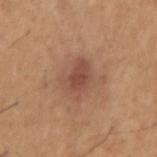{
  "image": {
    "source": "total-body photography crop",
    "field_of_view_mm": 15
  },
  "automated_metrics": {
    "cielab_L": 49,
    "cielab_a": 22,
    "cielab_b": 28,
    "vs_skin_darker_L": 9.0,
    "vs_skin_contrast_norm": 7.0,
    "border_irregularity_0_10": 3.5,
    "peripheral_color_asymmetry": 1.0
  },
  "lesion_size": {
    "long_diameter_mm_approx": 4.0
  },
  "lighting": "white-light",
  "site": "right upper arm",
  "patient": {
    "sex": "male",
    "age_approx": 65
  }
}